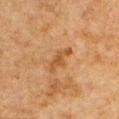Imaged during a routine full-body skin examination; the lesion was not biopsied and no histopathology is available. The patient is a male aged 78 to 82. Measured at roughly 4 mm in maximum diameter. A 15 mm close-up tile from a total-body photography series done for melanoma screening. Imaged with cross-polarized lighting. Located on the chest.